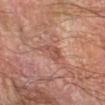Imaged during a routine full-body skin examination; the lesion was not biopsied and no histopathology is available. A male subject aged 68–72. From the arm. A 15 mm crop from a total-body photograph taken for skin-cancer surveillance.